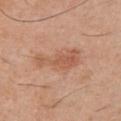{"lesion_size": {"long_diameter_mm_approx": 5.5}, "patient": {"sex": "male", "age_approx": 30}, "lighting": "white-light", "image": {"source": "total-body photography crop", "field_of_view_mm": 15}, "site": "chest"}